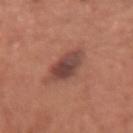Clinical impression: Imaged during a routine full-body skin examination; the lesion was not biopsied and no histopathology is available. Image and clinical context: A female patient, aged 63 to 67. Cropped from a total-body skin-imaging series; the visible field is about 15 mm. The lesion is located on the arm. Longest diameter approximately 3.5 mm.